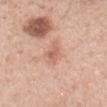biopsy_status: not biopsied; imaged during a skin examination